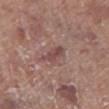Q: Is there a histopathology result?
A: imaged on a skin check; not biopsied
Q: How was the tile lit?
A: white-light illumination
Q: Who is the patient?
A: male, aged 68 to 72
Q: What did automated image analysis measure?
A: a border-irregularity rating of about 4.5/10, a color-variation rating of about 2/10, and radial color variation of about 0.5
Q: What is the imaging modality?
A: ~15 mm crop, total-body skin-cancer survey
Q: What is the anatomic site?
A: the left lower leg
Q: How large is the lesion?
A: ≈3.5 mm